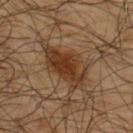Clinical impression:
The lesion was photographed on a routine skin check and not biopsied; there is no pathology result.
Image and clinical context:
On the upper back. The lesion's longest dimension is about 6.5 mm. The total-body-photography lesion software estimated a footprint of about 16 mm², a shape eccentricity near 0.8, and a symmetry-axis asymmetry near 0.35. It also reported about 10 CIELAB-L* units darker than the surrounding skin and a normalized border contrast of about 9. The patient is a male aged 63–67. A region of skin cropped from a whole-body photographic capture, roughly 15 mm wide. This is a cross-polarized tile.